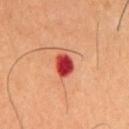This lesion was catalogued during total-body skin photography and was not selected for biopsy. A 15 mm crop from a total-body photograph taken for skin-cancer surveillance. The lesion is located on the chest. Approximately 3.5 mm at its widest. A male subject, aged approximately 60. Captured under cross-polarized illumination.